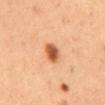The lesion was tiled from a total-body skin photograph and was not biopsied. The subject is a male about 50 years old. Measured at roughly 3 mm in maximum diameter. A roughly 15 mm field-of-view crop from a total-body skin photograph. This is a cross-polarized tile. Located on the abdomen.